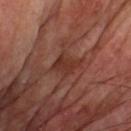This lesion was catalogued during total-body skin photography and was not selected for biopsy.
The patient is a male approximately 65 years of age.
Longest diameter approximately 3 mm.
Cropped from a whole-body photographic skin survey; the tile spans about 15 mm.
The tile uses cross-polarized illumination.
From the chest.